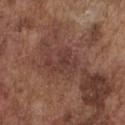Assessment: Recorded during total-body skin imaging; not selected for excision or biopsy. Background: Longest diameter approximately 4 mm. Located on the front of the torso. The total-body-photography lesion software estimated border irregularity of about 5.5 on a 0–10 scale, a color-variation rating of about 2.5/10, and peripheral color asymmetry of about 1. The software also gave a lesion-detection confidence of about 100/100. A male subject, approximately 75 years of age. A 15 mm close-up extracted from a 3D total-body photography capture.